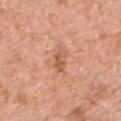| key | value |
|---|---|
| workup | imaged on a skin check; not biopsied |